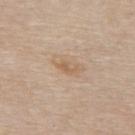Imaged during a routine full-body skin examination; the lesion was not biopsied and no histopathology is available.
Located on the upper back.
Imaged with white-light lighting.
The patient is a male aged 83 or older.
Cropped from a total-body skin-imaging series; the visible field is about 15 mm.